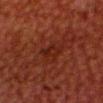| feature | finding |
|---|---|
| biopsy status | imaged on a skin check; not biopsied |
| illumination | cross-polarized illumination |
| size | ≈3 mm |
| subject | male, about 60 years old |
| acquisition | total-body-photography crop, ~15 mm field of view |
| location | the head or neck |
| image-analysis metrics | a lesion area of about 4 mm², a shape eccentricity near 0.8, and a symmetry-axis asymmetry near 0.25; roughly 4 lightness units darker than nearby skin and a normalized border contrast of about 5; a classifier nevus-likeness of about 0/100 and lesion-presence confidence of about 100/100 |